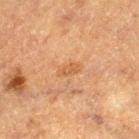The lesion was tiled from a total-body skin photograph and was not biopsied.
A 15 mm close-up extracted from a 3D total-body photography capture.
A male subject in their mid-70s.
On the left thigh.
Longest diameter approximately 2.5 mm.
Captured under cross-polarized illumination.